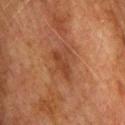Clinical impression: This lesion was catalogued during total-body skin photography and was not selected for biopsy. Clinical summary: On the upper back. A male subject approximately 75 years of age. A region of skin cropped from a whole-body photographic capture, roughly 15 mm wide.